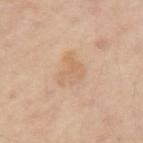Context: The subject is a female aged around 45. The total-body-photography lesion software estimated a footprint of about 8 mm² and two-axis asymmetry of about 0.45. The software also gave an average lesion color of about L≈65 a*≈17 b*≈34 (CIELAB) and roughly 6 lightness units darker than nearby skin. The analysis additionally found border irregularity of about 4.5 on a 0–10 scale, a within-lesion color-variation index near 3/10, and peripheral color asymmetry of about 1. On the arm. The tile uses cross-polarized illumination. A 15 mm close-up tile from a total-body photography series done for melanoma screening.